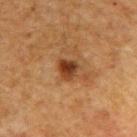Impression: Part of a total-body skin-imaging series; this lesion was reviewed on a skin check and was not flagged for biopsy. Acquisition and patient details: Located on the upper back. A male patient aged approximately 50. This is a cross-polarized tile. The lesion-visualizer software estimated a lesion color around L≈32 a*≈20 b*≈30 in CIELAB, a lesion–skin lightness drop of about 11, and a normalized lesion–skin contrast near 10.5. The analysis additionally found a border-irregularity index near 2.5/10, a within-lesion color-variation index near 5/10, and a peripheral color-asymmetry measure near 1.5. The software also gave an automated nevus-likeness rating near 90 out of 100 and a lesion-detection confidence of about 100/100. Approximately 3 mm at its widest. A lesion tile, about 15 mm wide, cut from a 3D total-body photograph.